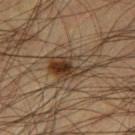{"biopsy_status": "not biopsied; imaged during a skin examination", "site": "left thigh", "lighting": "cross-polarized", "patient": {"sex": "male", "age_approx": 45}, "image": {"source": "total-body photography crop", "field_of_view_mm": 15}, "lesion_size": {"long_diameter_mm_approx": 5.5}}